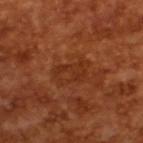Impression: The lesion was tiled from a total-body skin photograph and was not biopsied. Image and clinical context: A roughly 15 mm field-of-view crop from a total-body skin photograph. A male subject in their mid-60s. The tile uses cross-polarized illumination.